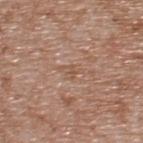Assessment:
Part of a total-body skin-imaging series; this lesion was reviewed on a skin check and was not flagged for biopsy.